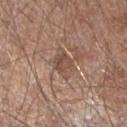– biopsy status · catalogued during a skin exam; not biopsied
– image source · ~15 mm tile from a whole-body skin photo
– size · ≈3 mm
– location · the arm
– patient · male, aged approximately 60
– automated metrics · a lesion area of about 5.5 mm² and an eccentricity of roughly 0.65; a border-irregularity index near 2/10, internal color variation of about 3.5 on a 0–10 scale, and a peripheral color-asymmetry measure near 1.5; a classifier nevus-likeness of about 0/100 and lesion-presence confidence of about 60/100